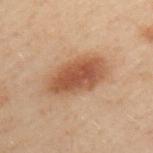<tbp_lesion>
<biopsy_status>not biopsied; imaged during a skin examination</biopsy_status>
<image>
  <source>total-body photography crop</source>
  <field_of_view_mm>15</field_of_view_mm>
</image>
<lesion_size>
  <long_diameter_mm_approx>7.0</long_diameter_mm_approx>
</lesion_size>
<patient>
  <sex>male</sex>
  <age_approx>50</age_approx>
</patient>
<automated_metrics>
  <cielab_L>43</cielab_L>
  <cielab_a>19</cielab_a>
  <cielab_b>28</cielab_b>
  <vs_skin_contrast_norm>9.0</vs_skin_contrast_norm>
  <border_irregularity_0_10>2.0</border_irregularity_0_10>
  <color_variation_0_10>4.0</color_variation_0_10>
  <peripheral_color_asymmetry>1.0</peripheral_color_asymmetry>
  <nevus_likeness_0_100>100</nevus_likeness_0_100>
  <lesion_detection_confidence_0_100>100</lesion_detection_confidence_0_100>
</automated_metrics>
<lighting>cross-polarized</lighting>
<site>left upper arm</site>
</tbp_lesion>